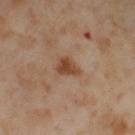follow-up: imaged on a skin check; not biopsied
TBP lesion metrics: a lesion area of about 5 mm² and a shape-asymmetry score of about 0.4 (0 = symmetric); a border-irregularity rating of about 3.5/10 and a within-lesion color-variation index near 3/10
subject: female, in their mid-50s
size: ≈3 mm
imaging modality: 15 mm crop, total-body photography
site: the left thigh
lighting: cross-polarized